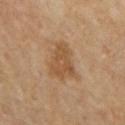Context: The lesion is located on the mid back. Captured under cross-polarized illumination. The lesion's longest dimension is about 4.5 mm. A 15 mm crop from a total-body photograph taken for skin-cancer surveillance. The patient is a male approximately 65 years of age.